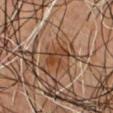Captured during whole-body skin photography for melanoma surveillance; the lesion was not biopsied.
About 3 mm across.
On the chest.
The lesion-visualizer software estimated an area of roughly 6 mm², a shape eccentricity near 0.65, and a shape-asymmetry score of about 0.35 (0 = symmetric). The analysis additionally found a detector confidence of about 90 out of 100 that the crop contains a lesion.
A male subject in their mid-40s.
Cropped from a total-body skin-imaging series; the visible field is about 15 mm.
The tile uses cross-polarized illumination.